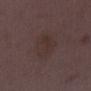{
  "biopsy_status": "not biopsied; imaged during a skin examination",
  "image": {
    "source": "total-body photography crop",
    "field_of_view_mm": 15
  },
  "patient": {
    "sex": "female",
    "age_approx": 30
  },
  "site": "right lower leg",
  "lesion_size": {
    "long_diameter_mm_approx": 4.5
  },
  "lighting": "white-light",
  "automated_metrics": {
    "vs_skin_contrast_norm": 5.0,
    "color_variation_0_10": 2.5,
    "nevus_likeness_0_100": 0,
    "lesion_detection_confidence_0_100": 100
  }
}